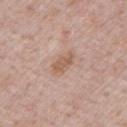notes = catalogued during a skin exam; not biopsied | patient = male, aged 53–57 | image source = 15 mm crop, total-body photography.